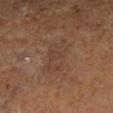notes: no biopsy performed (imaged during a skin exam); tile lighting: cross-polarized; image: 15 mm crop, total-body photography; site: the leg; subject: male, approximately 75 years of age.